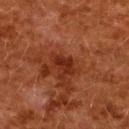Recorded during total-body skin imaging; not selected for excision or biopsy. An algorithmic analysis of the crop reported a lesion color around L≈23 a*≈25 b*≈27 in CIELAB, about 8 CIELAB-L* units darker than the surrounding skin, and a normalized lesion–skin contrast near 8. The software also gave border irregularity of about 3.5 on a 0–10 scale, a color-variation rating of about 1/10, and radial color variation of about 0.5. The tile uses cross-polarized illumination. The patient is a female about 50 years old. A region of skin cropped from a whole-body photographic capture, roughly 15 mm wide. From the upper back.